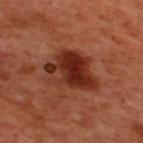Impression: The lesion was photographed on a routine skin check and not biopsied; there is no pathology result. Image and clinical context: A roughly 15 mm field-of-view crop from a total-body skin photograph. The tile uses cross-polarized illumination. The recorded lesion diameter is about 6 mm. The lesion is on the back. Automated tile analysis of the lesion measured border irregularity of about 4 on a 0–10 scale and peripheral color asymmetry of about 2.5. It also reported lesion-presence confidence of about 100/100. A male subject, aged approximately 50.